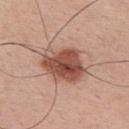{
  "biopsy_status": "not biopsied; imaged during a skin examination",
  "lighting": "white-light",
  "lesion_size": {
    "long_diameter_mm_approx": 5.5
  },
  "image": {
    "source": "total-body photography crop",
    "field_of_view_mm": 15
  },
  "patient": {
    "sex": "male",
    "age_approx": 50
  },
  "site": "upper back"
}